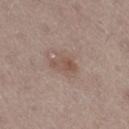- location: the right thigh
- subject: male, in their mid- to late 60s
- illumination: white-light illumination
- image: 15 mm crop, total-body photography
- size: ≈3.5 mm